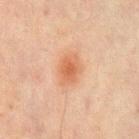biopsy status=total-body-photography surveillance lesion; no biopsy | lighting=cross-polarized illumination | image source=total-body-photography crop, ~15 mm field of view | subject=male, about 70 years old | location=the mid back | size=~3.5 mm (longest diameter) | image-analysis metrics=a border-irregularity index near 2/10 and radial color variation of about 0.5; a nevus-likeness score of about 90/100.